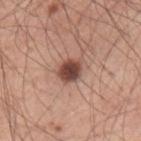workup = total-body-photography surveillance lesion; no biopsy
image source = 15 mm crop, total-body photography
subject = male, aged around 60
location = the arm